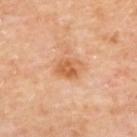Case summary:
- biopsy status: catalogued during a skin exam; not biopsied
- subject: female, aged around 60
- image: ~15 mm tile from a whole-body skin photo
- lesion diameter: about 3.5 mm
- site: the upper back
- tile lighting: cross-polarized